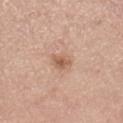The lesion was tiled from a total-body skin photograph and was not biopsied.
A 15 mm crop from a total-body photograph taken for skin-cancer surveillance.
Captured under white-light illumination.
A female patient, in their mid- to late 50s.
Measured at roughly 2.5 mm in maximum diameter.
The lesion is on the right lower leg.